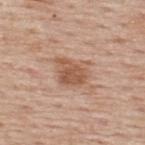The lesion was photographed on a routine skin check and not biopsied; there is no pathology result.
The lesion is located on the upper back.
A 15 mm crop from a total-body photograph taken for skin-cancer surveillance.
Imaged with white-light lighting.
A male subject, approximately 75 years of age.